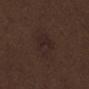biopsy status: no biopsy performed (imaged during a skin exam) | body site: the chest | image-analysis metrics: a lesion color around L≈23 a*≈13 b*≈16 in CIELAB and a normalized border contrast of about 6; border irregularity of about 3 on a 0–10 scale, a within-lesion color-variation index near 3/10, and a peripheral color-asymmetry measure near 1; an automated nevus-likeness rating near 10 out of 100 | imaging modality: ~15 mm tile from a whole-body skin photo | lesion size: about 5 mm | subject: male, aged 68 to 72 | lighting: white-light.